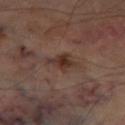| key | value |
|---|---|
| biopsy status | no biopsy performed (imaged during a skin exam) |
| patient | male, aged 68–72 |
| acquisition | ~15 mm tile from a whole-body skin photo |
| anatomic site | the left thigh |